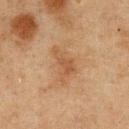workup: catalogued during a skin exam; not biopsied
image: 15 mm crop, total-body photography
anatomic site: the chest
patient: male, about 75 years old